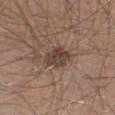Approximately 3.5 mm at its widest.
This is a white-light tile.
A male subject aged around 30.
This image is a 15 mm lesion crop taken from a total-body photograph.
Located on the right lower leg.